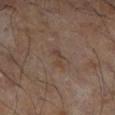No biopsy was performed on this lesion — it was imaged during a full skin examination and was not determined to be concerning.
A close-up tile cropped from a whole-body skin photograph, about 15 mm across.
The subject is a male about 55 years old.
Located on the leg.